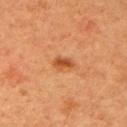workup: imaged on a skin check; not biopsied | tile lighting: cross-polarized | patient: female, aged approximately 40 | imaging modality: total-body-photography crop, ~15 mm field of view | body site: the right upper arm | size: about 2.5 mm.